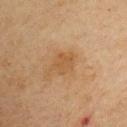Captured during whole-body skin photography for melanoma surveillance; the lesion was not biopsied.
On the chest.
A male patient in their mid- to late 60s.
A lesion tile, about 15 mm wide, cut from a 3D total-body photograph.